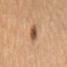{"biopsy_status": "not biopsied; imaged during a skin examination"}